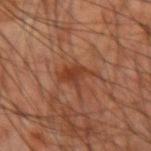The lesion was photographed on a routine skin check and not biopsied; there is no pathology result. A male subject in their mid-60s. Automated image analysis of the tile measured a lesion color around L≈33 a*≈22 b*≈28 in CIELAB, roughly 7 lightness units darker than nearby skin, and a normalized border contrast of about 7. The tile uses cross-polarized illumination. Cropped from a whole-body photographic skin survey; the tile spans about 15 mm. From the right forearm.